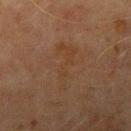<lesion>
<biopsy_status>not biopsied; imaged during a skin examination</biopsy_status>
<lighting>cross-polarized</lighting>
<site>right upper arm</site>
<patient>
  <sex>male</sex>
  <age_approx>70</age_approx>
</patient>
<lesion_size>
  <long_diameter_mm_approx>6.0</long_diameter_mm_approx>
</lesion_size>
<image>
  <source>total-body photography crop</source>
  <field_of_view_mm>15</field_of_view_mm>
</image>
</lesion>